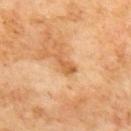follow-up=catalogued during a skin exam; not biopsied
automated lesion analysis=an average lesion color of about L≈59 a*≈25 b*≈42 (CIELAB) and about 10 CIELAB-L* units darker than the surrounding skin; border irregularity of about 4.5 on a 0–10 scale, a color-variation rating of about 1.5/10, and a peripheral color-asymmetry measure near 0.5; a nevus-likeness score of about 0/100
subject=male, aged 68–72
site=the back
lesion size=about 3 mm
lighting=cross-polarized illumination
imaging modality=total-body-photography crop, ~15 mm field of view